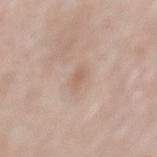Impression: Captured during whole-body skin photography for melanoma surveillance; the lesion was not biopsied. Clinical summary: From the mid back. Captured under white-light illumination. The patient is a male aged 53–57. The recorded lesion diameter is about 2.5 mm. Cropped from a total-body skin-imaging series; the visible field is about 15 mm.